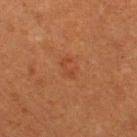Assessment:
This lesion was catalogued during total-body skin photography and was not selected for biopsy.
Clinical summary:
This image is a 15 mm lesion crop taken from a total-body photograph. About 2.5 mm across. Captured under cross-polarized illumination. A female patient, aged 53–57. Located on the right thigh. Automated tile analysis of the lesion measured a lesion color around L≈38 a*≈24 b*≈33 in CIELAB, a lesion–skin lightness drop of about 5, and a lesion-to-skin contrast of about 4.5 (normalized; higher = more distinct). The analysis additionally found a border-irregularity rating of about 6/10 and a peripheral color-asymmetry measure near 0.